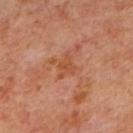Clinical impression: Captured during whole-body skin photography for melanoma surveillance; the lesion was not biopsied. Clinical summary: The lesion is on the upper back. This is a cross-polarized tile. Measured at roughly 3 mm in maximum diameter. A 15 mm crop from a total-body photograph taken for skin-cancer surveillance. A subject approximately 65 years of age.